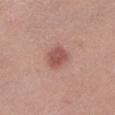lesion size — ~2.5 mm (longest diameter)
location — the left lower leg
patient — female, approximately 55 years of age
lighting — white-light illumination
image — 15 mm crop, total-body photography
image-analysis metrics — an area of roughly 7 mm², an outline eccentricity of about 0.35 (0 = round, 1 = elongated), and a symmetry-axis asymmetry near 0.25; a lesion color around L≈53 a*≈25 b*≈25 in CIELAB and a lesion–skin lightness drop of about 11